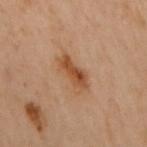Clinical impression:
This lesion was catalogued during total-body skin photography and was not selected for biopsy.
Background:
The lesion is on the upper back. A close-up tile cropped from a whole-body skin photograph, about 15 mm across. The lesion's longest dimension is about 4 mm. Automated image analysis of the tile measured a footprint of about 7.5 mm², an eccentricity of roughly 0.85, and a shape-asymmetry score of about 0.25 (0 = symmetric). It also reported an average lesion color of about L≈43 a*≈20 b*≈31 (CIELAB), roughly 9 lightness units darker than nearby skin, and a normalized lesion–skin contrast near 8. The software also gave border irregularity of about 3 on a 0–10 scale, a color-variation rating of about 4.5/10, and peripheral color asymmetry of about 1.5. A female subject, aged approximately 60. The tile uses cross-polarized illumination.